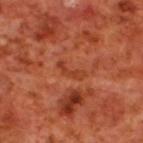The lesion was photographed on a routine skin check and not biopsied; there is no pathology result.
This is a cross-polarized tile.
A male patient, approximately 70 years of age.
On the back.
Automated image analysis of the tile measured an outline eccentricity of about 0.95 (0 = round, 1 = elongated). The analysis additionally found a border-irregularity index near 5.5/10.
The lesion's longest dimension is about 3.5 mm.
This image is a 15 mm lesion crop taken from a total-body photograph.